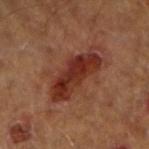Clinical impression:
The lesion was photographed on a routine skin check and not biopsied; there is no pathology result.
Background:
A 15 mm crop from a total-body photograph taken for skin-cancer surveillance. Measured at roughly 7 mm in maximum diameter. Automated image analysis of the tile measured a footprint of about 16 mm², a shape eccentricity near 0.9, and a shape-asymmetry score of about 0.3 (0 = symmetric). The software also gave border irregularity of about 4 on a 0–10 scale, a within-lesion color-variation index near 4.5/10, and peripheral color asymmetry of about 1.5. The lesion is located on the right forearm. A male subject aged around 60.